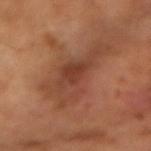<case>
<image>
  <source>total-body photography crop</source>
  <field_of_view_mm>15</field_of_view_mm>
</image>
<patient>
  <sex>male</sex>
  <age_approx>65</age_approx>
</patient>
</case>